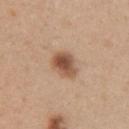{
  "biopsy_status": "not biopsied; imaged during a skin examination",
  "lesion_size": {
    "long_diameter_mm_approx": 3.5
  },
  "image": {
    "source": "total-body photography crop",
    "field_of_view_mm": 15
  },
  "automated_metrics": {
    "border_irregularity_0_10": 2.0,
    "color_variation_0_10": 6.0,
    "peripheral_color_asymmetry": 1.5,
    "lesion_detection_confidence_0_100": 100
  },
  "lighting": "white-light",
  "site": "chest",
  "patient": {
    "sex": "female",
    "age_approx": 30
  }
}